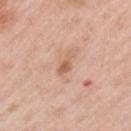biopsy status: total-body-photography surveillance lesion; no biopsy | illumination: white-light | image: 15 mm crop, total-body photography | lesion size: about 3 mm | subject: female, aged around 50 | site: the left upper arm.